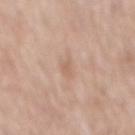Assessment: The lesion was photographed on a routine skin check and not biopsied; there is no pathology result. Acquisition and patient details: Measured at roughly 2.5 mm in maximum diameter. A male subject aged around 80. A close-up tile cropped from a whole-body skin photograph, about 15 mm across. Captured under white-light illumination. On the mid back.